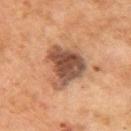Assessment:
This lesion was catalogued during total-body skin photography and was not selected for biopsy.
Clinical summary:
An algorithmic analysis of the crop reported border irregularity of about 4 on a 0–10 scale, internal color variation of about 4.5 on a 0–10 scale, and radial color variation of about 1.5. The software also gave an automated nevus-likeness rating near 45 out of 100. This is a cross-polarized tile. The lesion is on the left upper arm. A female subject about 60 years old. The lesion's longest dimension is about 6 mm. A region of skin cropped from a whole-body photographic capture, roughly 15 mm wide.